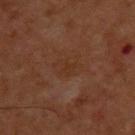Imaged during a routine full-body skin examination; the lesion was not biopsied and no histopathology is available.